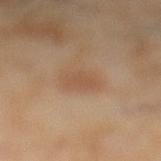{"biopsy_status": "not biopsied; imaged during a skin examination", "lesion_size": {"long_diameter_mm_approx": 3.5}, "lighting": "cross-polarized", "patient": {"sex": "female", "age_approx": 60}, "site": "left lower leg", "image": {"source": "total-body photography crop", "field_of_view_mm": 15}}